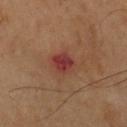<lesion>
<biopsy_status>not biopsied; imaged during a skin examination</biopsy_status>
<site>chest</site>
<patient>
  <sex>male</sex>
  <age_approx>65</age_approx>
</patient>
<lighting>cross-polarized</lighting>
<image>
  <source>total-body photography crop</source>
  <field_of_view_mm>15</field_of_view_mm>
</image>
</lesion>